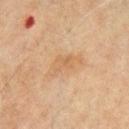Q: Is there a histopathology result?
A: no biopsy performed (imaged during a skin exam)
Q: Lesion location?
A: the chest
Q: What are the patient's age and sex?
A: male, in their 70s
Q: How was this image acquired?
A: ~15 mm crop, total-body skin-cancer survey
Q: What did automated image analysis measure?
A: a mean CIELAB color near L≈51 a*≈16 b*≈31, about 6 CIELAB-L* units darker than the surrounding skin, and a normalized lesion–skin contrast near 5; a border-irregularity rating of about 5/10, a within-lesion color-variation index near 2/10, and a peripheral color-asymmetry measure near 0.5
Q: What lighting was used for the tile?
A: cross-polarized illumination
Q: What is the lesion's diameter?
A: ~4.5 mm (longest diameter)